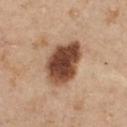Notes:
• workup · total-body-photography surveillance lesion; no biopsy
• lighting · white-light illumination
• size · about 6 mm
• patient · female, aged approximately 45
• acquisition · 15 mm crop, total-body photography
• body site · the chest